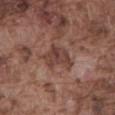Imaged during a routine full-body skin examination; the lesion was not biopsied and no histopathology is available.
On the abdomen.
A region of skin cropped from a whole-body photographic capture, roughly 15 mm wide.
The total-body-photography lesion software estimated a lesion area of about 8.5 mm², a shape eccentricity near 0.6, and a shape-asymmetry score of about 0.4 (0 = symmetric). It also reported a mean CIELAB color near L≈41 a*≈19 b*≈24, about 9 CIELAB-L* units darker than the surrounding skin, and a normalized lesion–skin contrast near 7.5. And it measured a lesion-detection confidence of about 100/100.
Measured at roughly 4 mm in maximum diameter.
This is a white-light tile.
A male patient, roughly 75 years of age.